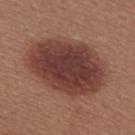<case>
  <biopsy_status>not biopsied; imaged during a skin examination</biopsy_status>
  <patient>
    <sex>female</sex>
    <age_approx>25</age_approx>
  </patient>
  <image>
    <source>total-body photography crop</source>
    <field_of_view_mm>15</field_of_view_mm>
  </image>
  <site>upper back</site>
  <lesion_size>
    <long_diameter_mm_approx>9.0</long_diameter_mm_approx>
  </lesion_size>
</case>